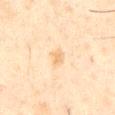workup: total-body-photography surveillance lesion; no biopsy
anatomic site: the abdomen
patient: male, aged 43 to 47
lighting: cross-polarized illumination
image: ~15 mm crop, total-body skin-cancer survey